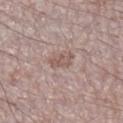Acquisition and patient details:
A close-up tile cropped from a whole-body skin photograph, about 15 mm across. A male patient, aged around 50. On the leg.